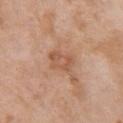| feature | finding |
|---|---|
| notes | total-body-photography surveillance lesion; no biopsy |
| tile lighting | white-light illumination |
| image-analysis metrics | a lesion area of about 5.5 mm² and an eccentricity of roughly 0.75; a lesion color around L≈55 a*≈22 b*≈33 in CIELAB, a lesion–skin lightness drop of about 8, and a normalized lesion–skin contrast near 5.5; a classifier nevus-likeness of about 0/100 and a lesion-detection confidence of about 100/100 |
| size | ~3 mm (longest diameter) |
| location | the chest |
| subject | female, about 70 years old |
| acquisition | ~15 mm tile from a whole-body skin photo |